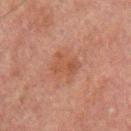A lesion tile, about 15 mm wide, cut from a 3D total-body photograph. This is a cross-polarized tile. An algorithmic analysis of the crop reported a mean CIELAB color near L≈38 a*≈20 b*≈26 and a lesion–skin lightness drop of about 5. The software also gave a color-variation rating of about 2.5/10. A male subject, aged approximately 60. The lesion is on the left upper arm. Approximately 3.5 mm at its widest.